Automated tile analysis of the lesion measured an area of roughly 16 mm² and an eccentricity of roughly 0.9. The software also gave a mean CIELAB color near L≈57 a*≈22 b*≈31, a lesion–skin lightness drop of about 8, and a normalized border contrast of about 7. And it measured a border-irregularity rating of about 6.5/10, a color-variation rating of about 2.5/10, and peripheral color asymmetry of about 1. And it measured a classifier nevus-likeness of about 0/100 and a detector confidence of about 100 out of 100 that the crop contains a lesion; a female patient, approximately 60 years of age; approximately 7.5 mm at its widest; a 15 mm crop from a total-body photograph taken for skin-cancer surveillance; located on the head or neck; the tile uses white-light illumination: 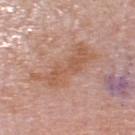Biopsy histopathology demonstrated a solar lentigo (benign).